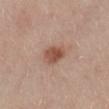biopsy_status: not biopsied; imaged during a skin examination
image:
  source: total-body photography crop
  field_of_view_mm: 15
automated_metrics:
  area_mm2_approx: 6.5
  shape_asymmetry: 0.15
  cielab_L: 52
  cielab_a: 22
  cielab_b: 28
  vs_skin_darker_L: 11.0
  vs_skin_contrast_norm: 8.5
  border_irregularity_0_10: 1.5
  color_variation_0_10: 4.0
  peripheral_color_asymmetry: 1.5
patient:
  sex: female
  age_approx: 55
lighting: white-light
site: right lower leg